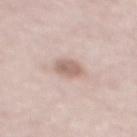Notes:
• follow-up · no biopsy performed (imaged during a skin exam)
• automated lesion analysis · a border-irregularity rating of about 1.5/10, a color-variation rating of about 2/10, and peripheral color asymmetry of about 1
• subject · female, in their 50s
• lighting · white-light illumination
• diameter · ≈3 mm
• site · the mid back
• image · 15 mm crop, total-body photography